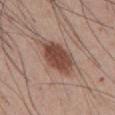Assessment: No biopsy was performed on this lesion — it was imaged during a full skin examination and was not determined to be concerning. Acquisition and patient details: This is a white-light tile. The patient is a male aged 43 to 47. On the abdomen. A 15 mm crop from a total-body photograph taken for skin-cancer surveillance.